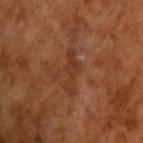Q: Was a biopsy performed?
A: no biopsy performed (imaged during a skin exam)
Q: What did automated image analysis measure?
A: a mean CIELAB color near L≈35 a*≈24 b*≈32, roughly 6 lightness units darker than nearby skin, and a normalized border contrast of about 5.5; border irregularity of about 6.5 on a 0–10 scale and a color-variation rating of about 1/10; an automated nevus-likeness rating near 0 out of 100
Q: What lighting was used for the tile?
A: cross-polarized illumination
Q: What is the imaging modality?
A: ~15 mm crop, total-body skin-cancer survey
Q: What are the patient's age and sex?
A: male, in their mid-60s
Q: What is the lesion's diameter?
A: about 5 mm